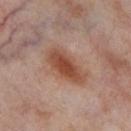Assessment:
Captured during whole-body skin photography for melanoma surveillance; the lesion was not biopsied.
Image and clinical context:
The lesion-visualizer software estimated a border-irregularity rating of about 2/10, internal color variation of about 4 on a 0–10 scale, and peripheral color asymmetry of about 1. Cropped from a total-body skin-imaging series; the visible field is about 15 mm. A female subject, aged around 55. The tile uses cross-polarized illumination. The lesion is on the left thigh.